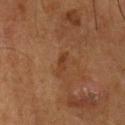Findings:
– follow-up: imaged on a skin check; not biopsied